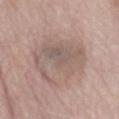Assessment:
Part of a total-body skin-imaging series; this lesion was reviewed on a skin check and was not flagged for biopsy.
Clinical summary:
Longest diameter approximately 7.5 mm. The lesion is located on the abdomen. This image is a 15 mm lesion crop taken from a total-body photograph. The subject is a male aged 68–72. This is a white-light tile.